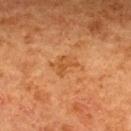Recorded during total-body skin imaging; not selected for excision or biopsy. A 15 mm close-up tile from a total-body photography series done for melanoma screening. Imaged with cross-polarized lighting. A female subject approximately 50 years of age. From the upper back.